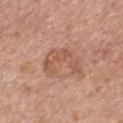Part of a total-body skin-imaging series; this lesion was reviewed on a skin check and was not flagged for biopsy.
A region of skin cropped from a whole-body photographic capture, roughly 15 mm wide.
Automated image analysis of the tile measured a lesion area of about 11 mm² and a symmetry-axis asymmetry near 0.6. And it measured a detector confidence of about 100 out of 100 that the crop contains a lesion.
Imaged with white-light lighting.
A male patient, about 60 years old.
Located on the chest.